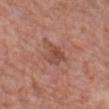This lesion was catalogued during total-body skin photography and was not selected for biopsy. This image is a 15 mm lesion crop taken from a total-body photograph. A male subject aged around 60. The recorded lesion diameter is about 3.5 mm. The lesion is on the chest.